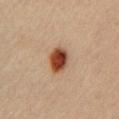Impression:
The lesion was photographed on a routine skin check and not biopsied; there is no pathology result.
Background:
Imaged with cross-polarized lighting. Automated tile analysis of the lesion measured a normalized lesion–skin contrast near 13.5. A close-up tile cropped from a whole-body skin photograph, about 15 mm across. A female subject aged around 45. Located on the front of the torso.